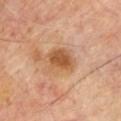Recorded during total-body skin imaging; not selected for excision or biopsy.
About 4 mm across.
A male patient, aged 68 to 72.
The total-body-photography lesion software estimated a lesion color around L≈54 a*≈22 b*≈37 in CIELAB and a normalized border contrast of about 8. The software also gave a border-irregularity index near 3/10, a within-lesion color-variation index near 3/10, and a peripheral color-asymmetry measure near 1. And it measured a nevus-likeness score of about 60/100 and a lesion-detection confidence of about 100/100.
Located on the chest.
A region of skin cropped from a whole-body photographic capture, roughly 15 mm wide.